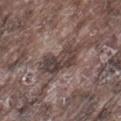Clinical impression:
The lesion was tiled from a total-body skin photograph and was not biopsied.
Image and clinical context:
A 15 mm close-up extracted from a 3D total-body photography capture. From the leg. The patient is a male aged approximately 75.